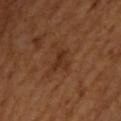Assessment: Imaged during a routine full-body skin examination; the lesion was not biopsied and no histopathology is available. Context: Longest diameter approximately 3 mm. On the back. This is a cross-polarized tile. A roughly 15 mm field-of-view crop from a total-body skin photograph. A male patient, approximately 65 years of age.